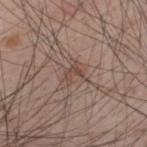Notes:
- notes — imaged on a skin check; not biopsied
- lesion diameter — about 2.5 mm
- subject — male, aged 33 to 37
- body site — the back
- image source — ~15 mm tile from a whole-body skin photo
- lighting — white-light